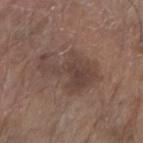This lesion was catalogued during total-body skin photography and was not selected for biopsy.
The subject is a male aged 63–67.
Measured at roughly 7 mm in maximum diameter.
A roughly 15 mm field-of-view crop from a total-body skin photograph.
Captured under white-light illumination.
An algorithmic analysis of the crop reported a lesion area of about 18 mm², an outline eccentricity of about 0.85 (0 = round, 1 = elongated), and two-axis asymmetry of about 0.55. The analysis additionally found border irregularity of about 8 on a 0–10 scale, a color-variation rating of about 3/10, and a peripheral color-asymmetry measure near 1. It also reported an automated nevus-likeness rating near 0 out of 100 and a detector confidence of about 100 out of 100 that the crop contains a lesion.
The lesion is on the right forearm.